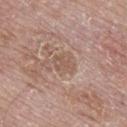| field | value |
|---|---|
| follow-up | catalogued during a skin exam; not biopsied |
| acquisition | total-body-photography crop, ~15 mm field of view |
| body site | the mid back |
| lighting | white-light |
| size | about 3 mm |
| subject | male, aged approximately 80 |